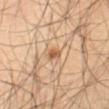follow-up — no biopsy performed (imaged during a skin exam) | lighting — cross-polarized illumination | acquisition — ~15 mm tile from a whole-body skin photo | lesion diameter — about 2 mm | subject — male, approximately 55 years of age | automated metrics — an automated nevus-likeness rating near 85 out of 100 and lesion-presence confidence of about 100/100 | anatomic site — the abdomen.